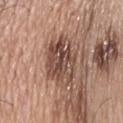{
  "biopsy_status": "not biopsied; imaged during a skin examination",
  "patient": {
    "sex": "male",
    "age_approx": 80
  },
  "site": "head or neck",
  "automated_metrics": {
    "eccentricity": 0.85,
    "shape_asymmetry": 0.2,
    "cielab_L": 48,
    "cielab_a": 19,
    "cielab_b": 26,
    "vs_skin_contrast_norm": 9.5,
    "border_irregularity_0_10": 3.5,
    "peripheral_color_asymmetry": 3.5
  },
  "lighting": "white-light",
  "lesion_size": {
    "long_diameter_mm_approx": 7.5
  },
  "image": {
    "source": "total-body photography crop",
    "field_of_view_mm": 15
  }
}